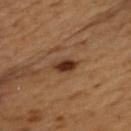| feature | finding |
|---|---|
| biopsy status | total-body-photography surveillance lesion; no biopsy |
| subject | male, about 55 years old |
| tile lighting | cross-polarized illumination |
| location | the chest |
| imaging modality | total-body-photography crop, ~15 mm field of view |
| size | ~2.5 mm (longest diameter) |
| TBP lesion metrics | an automated nevus-likeness rating near 95 out of 100 and a lesion-detection confidence of about 100/100 |